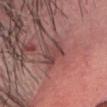Findings:
* biopsy status — imaged on a skin check; not biopsied
* patient — male, approximately 60 years of age
* lesion diameter — about 3.5 mm
* imaging modality — ~15 mm tile from a whole-body skin photo
* lighting — white-light illumination
* body site — the head or neck
* automated lesion analysis — an average lesion color of about L≈44 a*≈23 b*≈20 (CIELAB), a lesion–skin lightness drop of about 8, and a normalized lesion–skin contrast near 6.5